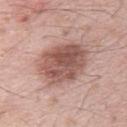This is a white-light tile. The lesion-visualizer software estimated roughly 14 lightness units darker than nearby skin and a normalized lesion–skin contrast near 9. It also reported a classifier nevus-likeness of about 25/100. A male patient aged 23–27. A 15 mm close-up extracted from a 3D total-body photography capture. The lesion is located on the back.